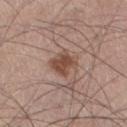Acquisition and patient details:
A male subject, approximately 45 years of age. An algorithmic analysis of the crop reported a footprint of about 6.5 mm² and a symmetry-axis asymmetry near 0.2. The analysis additionally found a mean CIELAB color near L≈48 a*≈20 b*≈27, about 11 CIELAB-L* units darker than the surrounding skin, and a normalized border contrast of about 8.5. It also reported a border-irregularity index near 2.5/10, a color-variation rating of about 2/10, and a peripheral color-asymmetry measure near 1. And it measured a classifier nevus-likeness of about 90/100 and lesion-presence confidence of about 100/100. The lesion is located on the right thigh. A 15 mm close-up tile from a total-body photography series done for melanoma screening. Measured at roughly 3 mm in maximum diameter. Imaged with white-light lighting.